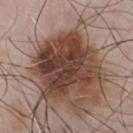workup: total-body-photography surveillance lesion; no biopsy
illumination: white-light illumination
image: total-body-photography crop, ~15 mm field of view
diameter: ≈7 mm
body site: the chest
subject: male, in their mid-50s
image-analysis metrics: a mean CIELAB color near L≈41 a*≈19 b*≈24, a lesion–skin lightness drop of about 15, and a normalized lesion–skin contrast near 12; border irregularity of about 3.5 on a 0–10 scale and internal color variation of about 6.5 on a 0–10 scale